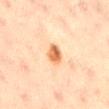biopsy_status: not biopsied; imaged during a skin examination
site: lower back
patient:
  sex: female
  age_approx: 20
lesion_size:
  long_diameter_mm_approx: 3.0
lighting: cross-polarized
image:
  source: total-body photography crop
  field_of_view_mm: 15
automated_metrics:
  area_mm2_approx: 5.0
  eccentricity: 0.75
  shape_asymmetry: 0.15
  vs_skin_darker_L: 13.0
  vs_skin_contrast_norm: 9.5
  nevus_likeness_0_100: 100
  lesion_detection_confidence_0_100: 100